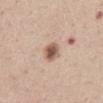Clinical impression: Part of a total-body skin-imaging series; this lesion was reviewed on a skin check and was not flagged for biopsy. Image and clinical context: The lesion is located on the front of the torso. Imaged with white-light lighting. This image is a 15 mm lesion crop taken from a total-body photograph. Longest diameter approximately 3 mm. A female patient aged approximately 45.